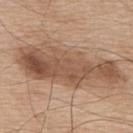notes: no biopsy performed (imaged during a skin exam) | site: the back | patient: male, aged 73 to 77 | lighting: white-light illumination | image: ~15 mm tile from a whole-body skin photo.